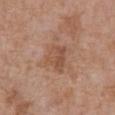No biopsy was performed on this lesion — it was imaged during a full skin examination and was not determined to be concerning. A male patient aged around 70. Automated image analysis of the tile measured an average lesion color of about L≈51 a*≈21 b*≈30 (CIELAB), roughly 8 lightness units darker than nearby skin, and a lesion-to-skin contrast of about 6 (normalized; higher = more distinct). It also reported a border-irregularity index near 3/10, a color-variation rating of about 4/10, and peripheral color asymmetry of about 1.5. The analysis additionally found an automated nevus-likeness rating near 0 out of 100 and a detector confidence of about 100 out of 100 that the crop contains a lesion. Cropped from a whole-body photographic skin survey; the tile spans about 15 mm. Imaged with white-light lighting. The lesion's longest dimension is about 3.5 mm. The lesion is on the abdomen.